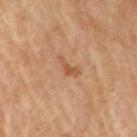acquisition: total-body-photography crop, ~15 mm field of view
automated metrics: a lesion area of about 3.5 mm², an outline eccentricity of about 0.9 (0 = round, 1 = elongated), and two-axis asymmetry of about 0.5; a classifier nevus-likeness of about 5/100 and a lesion-detection confidence of about 100/100
site: the arm
subject: male, about 70 years old
diameter: about 3 mm
illumination: cross-polarized illumination